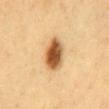imaging modality: ~15 mm tile from a whole-body skin photo | size: ≈4.5 mm | TBP lesion metrics: a nevus-likeness score of about 100/100 | patient: male, aged 58–62 | illumination: cross-polarized | site: the chest.